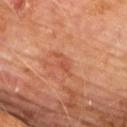Image and clinical context:
The lesion is located on the upper back. The recorded lesion diameter is about 3.5 mm. The subject is a male approximately 60 years of age. This is a cross-polarized tile. A lesion tile, about 15 mm wide, cut from a 3D total-body photograph.